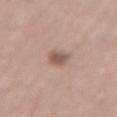Q: Was a biopsy performed?
A: imaged on a skin check; not biopsied
Q: What kind of image is this?
A: ~15 mm tile from a whole-body skin photo
Q: What is the lesion's diameter?
A: ≈3 mm
Q: Patient demographics?
A: male, roughly 55 years of age
Q: How was the tile lit?
A: white-light